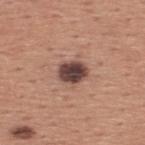{"biopsy_status": "not biopsied; imaged during a skin examination", "automated_metrics": {"cielab_L": 43, "cielab_a": 19, "cielab_b": 21, "vs_skin_darker_L": 18.0, "color_variation_0_10": 4.5, "peripheral_color_asymmetry": 1.5, "nevus_likeness_0_100": 25, "lesion_detection_confidence_0_100": 100}, "site": "upper back", "lesion_size": {"long_diameter_mm_approx": 3.5}, "image": {"source": "total-body photography crop", "field_of_view_mm": 15}, "patient": {"sex": "male", "age_approx": 45}}